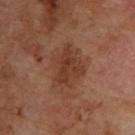Assessment: No biopsy was performed on this lesion — it was imaged during a full skin examination and was not determined to be concerning. Background: The patient is a male aged approximately 70. On the upper back. Cropped from a whole-body photographic skin survey; the tile spans about 15 mm.